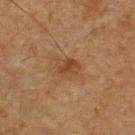The lesion's longest dimension is about 3 mm. Captured under cross-polarized illumination. Cropped from a total-body skin-imaging series; the visible field is about 15 mm. On the front of the torso. The patient is a male about 65 years old.